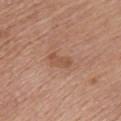Clinical impression: No biopsy was performed on this lesion — it was imaged during a full skin examination and was not determined to be concerning. Clinical summary: The recorded lesion diameter is about 3 mm. An algorithmic analysis of the crop reported a border-irregularity index near 3.5/10 and internal color variation of about 2 on a 0–10 scale. The analysis additionally found a classifier nevus-likeness of about 5/100 and lesion-presence confidence of about 100/100. Captured under white-light illumination. A male subject approximately 55 years of age. The lesion is located on the chest. Cropped from a whole-body photographic skin survey; the tile spans about 15 mm.